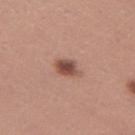Clinical impression: The lesion was tiled from a total-body skin photograph and was not biopsied. Background: A 15 mm close-up tile from a total-body photography series done for melanoma screening. Automated image analysis of the tile measured an area of roughly 4.5 mm², an outline eccentricity of about 0.75 (0 = round, 1 = elongated), and two-axis asymmetry of about 0.25. It also reported a lesion color around L≈49 a*≈22 b*≈26 in CIELAB and about 14 CIELAB-L* units darker than the surrounding skin. The lesion is on the left thigh. The subject is a female aged around 30. The recorded lesion diameter is about 3 mm.